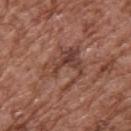Captured during whole-body skin photography for melanoma surveillance; the lesion was not biopsied.
The lesion-visualizer software estimated an area of roughly 14 mm², an eccentricity of roughly 0.55, and two-axis asymmetry of about 0.65. The analysis additionally found an average lesion color of about L≈42 a*≈22 b*≈27 (CIELAB), roughly 8 lightness units darker than nearby skin, and a normalized lesion–skin contrast near 6.5. The analysis additionally found a border-irregularity rating of about 10/10, a color-variation rating of about 7.5/10, and a peripheral color-asymmetry measure near 2.5. And it measured a lesion-detection confidence of about 60/100.
The lesion is located on the upper back.
A male subject, aged around 75.
Longest diameter approximately 6 mm.
Cropped from a whole-body photographic skin survey; the tile spans about 15 mm.
Imaged with white-light lighting.